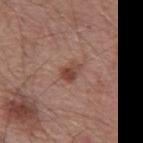Q: Was a biopsy performed?
A: catalogued during a skin exam; not biopsied
Q: What are the patient's age and sex?
A: male, approximately 55 years of age
Q: Automated lesion metrics?
A: an average lesion color of about L≈45 a*≈21 b*≈26 (CIELAB), roughly 9 lightness units darker than nearby skin, and a lesion-to-skin contrast of about 7.5 (normalized; higher = more distinct); a border-irregularity rating of about 2.5/10, internal color variation of about 5.5 on a 0–10 scale, and a peripheral color-asymmetry measure near 2
Q: What is the lesion's diameter?
A: about 2.5 mm
Q: What is the imaging modality?
A: ~15 mm crop, total-body skin-cancer survey
Q: Where on the body is the lesion?
A: the back